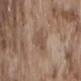{
  "biopsy_status": "not biopsied; imaged during a skin examination"
}